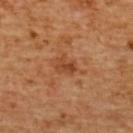{"biopsy_status": "not biopsied; imaged during a skin examination", "automated_metrics": {"cielab_L": 46, "cielab_a": 26, "cielab_b": 38, "vs_skin_darker_L": 9.0, "vs_skin_contrast_norm": 7.0, "nevus_likeness_0_100": 0, "lesion_detection_confidence_0_100": 100}, "image": {"source": "total-body photography crop", "field_of_view_mm": 15}, "lesion_size": {"long_diameter_mm_approx": 2.5}, "site": "upper back", "patient": {"sex": "female", "age_approx": 55}, "lighting": "cross-polarized"}